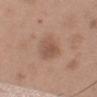Imaged during a routine full-body skin examination; the lesion was not biopsied and no histopathology is available. From the left upper arm. A male patient in their 50s. A 15 mm crop from a total-body photograph taken for skin-cancer surveillance. The tile uses white-light illumination. The lesion's longest dimension is about 4 mm.